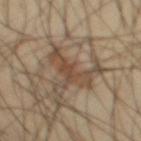Assessment:
Recorded during total-body skin imaging; not selected for excision or biopsy.
Image and clinical context:
The lesion is located on the front of the torso. The subject is a male approximately 40 years of age. About 4.5 mm across. Imaged with cross-polarized lighting. A 15 mm crop from a total-body photograph taken for skin-cancer surveillance.